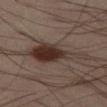Q: Was this lesion biopsied?
A: catalogued during a skin exam; not biopsied
Q: What lighting was used for the tile?
A: cross-polarized illumination
Q: Lesion size?
A: ≈7.5 mm
Q: What kind of image is this?
A: total-body-photography crop, ~15 mm field of view
Q: Automated lesion metrics?
A: a footprint of about 16 mm², a shape eccentricity near 0.85, and a symmetry-axis asymmetry near 0.4; an average lesion color of about L≈31 a*≈14 b*≈20 (CIELAB), a lesion–skin lightness drop of about 11, and a lesion-to-skin contrast of about 10.5 (normalized; higher = more distinct); a border-irregularity rating of about 5/10, internal color variation of about 6.5 on a 0–10 scale, and radial color variation of about 2
Q: What are the patient's age and sex?
A: male, about 40 years old
Q: Lesion location?
A: the leg